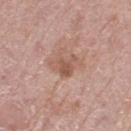Impression:
Imaged during a routine full-body skin examination; the lesion was not biopsied and no histopathology is available.
Image and clinical context:
Located on the right thigh. The subject is a female roughly 75 years of age. Cropped from a whole-body photographic skin survey; the tile spans about 15 mm. The tile uses white-light illumination.